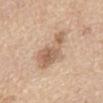This lesion was catalogued during total-body skin photography and was not selected for biopsy. The lesion is on the abdomen. An algorithmic analysis of the crop reported a mean CIELAB color near L≈61 a*≈17 b*≈31, about 11 CIELAB-L* units darker than the surrounding skin, and a normalized border contrast of about 7. The analysis additionally found an automated nevus-likeness rating near 15 out of 100 and a detector confidence of about 100 out of 100 that the crop contains a lesion. The subject is a male in their 70s. Cropped from a whole-body photographic skin survey; the tile spans about 15 mm.